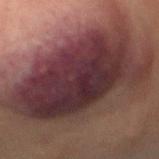Assessment: No biopsy was performed on this lesion — it was imaged during a full skin examination and was not determined to be concerning. Image and clinical context: A female subject aged around 60. The lesion is located on the chest. Imaged with cross-polarized lighting. A roughly 15 mm field-of-view crop from a total-body skin photograph. About 14.5 mm across.